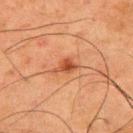The lesion was tiled from a total-body skin photograph and was not biopsied. On the upper back. Imaged with cross-polarized lighting. A male subject approximately 60 years of age. The recorded lesion diameter is about 3 mm. A 15 mm close-up tile from a total-body photography series done for melanoma screening.